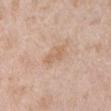This is a white-light tile. A male subject, aged 48–52. The total-body-photography lesion software estimated a mean CIELAB color near L≈63 a*≈18 b*≈32, a lesion–skin lightness drop of about 7, and a normalized lesion–skin contrast near 5.5. The recorded lesion diameter is about 3 mm. Located on the left upper arm. A 15 mm crop from a total-body photograph taken for skin-cancer surveillance.